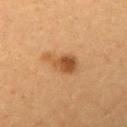notes — total-body-photography surveillance lesion; no biopsy
image — ~15 mm tile from a whole-body skin photo
subject — male, about 20 years old
lesion diameter — ~4 mm (longest diameter)
body site — the left upper arm